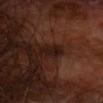| field | value |
|---|---|
| workup | imaged on a skin check; not biopsied |
| image | total-body-photography crop, ~15 mm field of view |
| tile lighting | cross-polarized |
| location | the head or neck |
| subject | male, in their 70s |
| size | about 3 mm |
| automated lesion analysis | a border-irregularity rating of about 3.5/10, internal color variation of about 1 on a 0–10 scale, and peripheral color asymmetry of about 0 |